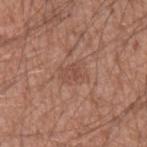Impression: Captured during whole-body skin photography for melanoma surveillance; the lesion was not biopsied. Acquisition and patient details: This is a white-light tile. From the arm. A male subject, in their 60s. Automated tile analysis of the lesion measured a lesion color around L≈49 a*≈22 b*≈27 in CIELAB, about 7 CIELAB-L* units darker than the surrounding skin, and a normalized lesion–skin contrast near 5. And it measured radial color variation of about 1. A 15 mm crop from a total-body photograph taken for skin-cancer surveillance.